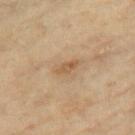The lesion was tiled from a total-body skin photograph and was not biopsied.
Located on the left thigh.
The recorded lesion diameter is about 2.5 mm.
This is a cross-polarized tile.
The patient is a female approximately 75 years of age.
Cropped from a whole-body photographic skin survey; the tile spans about 15 mm.